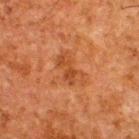notes: catalogued during a skin exam; not biopsied
body site: the upper back
lesion size: ≈4 mm
tile lighting: cross-polarized illumination
imaging modality: ~15 mm tile from a whole-body skin photo
subject: male, in their 60s